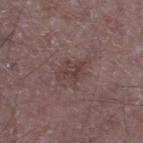| key | value |
|---|---|
| follow-up | total-body-photography surveillance lesion; no biopsy |
| image | ~15 mm tile from a whole-body skin photo |
| tile lighting | white-light illumination |
| body site | the leg |
| lesion size | ~3 mm (longest diameter) |
| patient | male, about 50 years old |